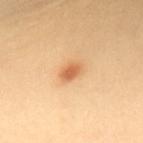This lesion was catalogued during total-body skin photography and was not selected for biopsy.
Located on the upper back.
Cropped from a whole-body photographic skin survey; the tile spans about 15 mm.
This is a cross-polarized tile.
A female patient aged around 40.